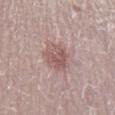Q: Was a biopsy performed?
A: no biopsy performed (imaged during a skin exam)
Q: Automated lesion metrics?
A: lesion-presence confidence of about 100/100
Q: What kind of image is this?
A: ~15 mm crop, total-body skin-cancer survey
Q: Where on the body is the lesion?
A: the left lower leg
Q: How was the tile lit?
A: white-light
Q: What is the lesion's diameter?
A: about 4 mm
Q: Patient demographics?
A: female, roughly 30 years of age